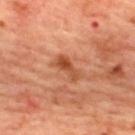Impression:
The lesion was photographed on a routine skin check and not biopsied; there is no pathology result.
Background:
An algorithmic analysis of the crop reported a mean CIELAB color near L≈50 a*≈28 b*≈38, about 10 CIELAB-L* units darker than the surrounding skin, and a normalized border contrast of about 8. And it measured a border-irregularity index near 4/10 and a within-lesion color-variation index near 3.5/10. The subject is a female about 45 years old. Captured under cross-polarized illumination. On the upper back. A roughly 15 mm field-of-view crop from a total-body skin photograph. Measured at roughly 3.5 mm in maximum diameter.